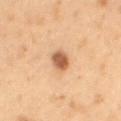The lesion was tiled from a total-body skin photograph and was not biopsied.
A lesion tile, about 15 mm wide, cut from a 3D total-body photograph.
On the mid back.
Captured under cross-polarized illumination.
Longest diameter approximately 2.5 mm.
The patient is a male in their mid- to late 50s.